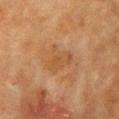No biopsy was performed on this lesion — it was imaged during a full skin examination and was not determined to be concerning.
A female subject, in their mid- to late 50s.
Captured under cross-polarized illumination.
The lesion is located on the chest.
A 15 mm close-up tile from a total-body photography series done for melanoma screening.
An algorithmic analysis of the crop reported a lesion area of about 7.5 mm², a shape eccentricity near 0.5, and a shape-asymmetry score of about 0.5 (0 = symmetric). It also reported an average lesion color of about L≈42 a*≈18 b*≈33 (CIELAB). And it measured an automated nevus-likeness rating near 0 out of 100 and a lesion-detection confidence of about 100/100.